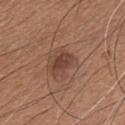follow-up: imaged on a skin check; not biopsied | patient: male, in their 60s | tile lighting: white-light | TBP lesion metrics: a lesion area of about 4.5 mm², an eccentricity of roughly 0.8, and a symmetry-axis asymmetry near 0.2; an automated nevus-likeness rating near 60 out of 100 | lesion size: ≈3 mm | acquisition: total-body-photography crop, ~15 mm field of view | anatomic site: the chest.